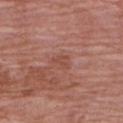Clinical impression:
No biopsy was performed on this lesion — it was imaged during a full skin examination and was not determined to be concerning.
Clinical summary:
Longest diameter approximately 2.5 mm. The lesion is on the arm. A close-up tile cropped from a whole-body skin photograph, about 15 mm across. The patient is a female aged around 70. The tile uses white-light illumination.